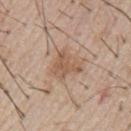follow-up: total-body-photography surveillance lesion; no biopsy
automated metrics: an average lesion color of about L≈56 a*≈18 b*≈30 (CIELAB) and a lesion-to-skin contrast of about 6.5 (normalized; higher = more distinct)
acquisition: ~15 mm tile from a whole-body skin photo
tile lighting: white-light illumination
patient: male, roughly 55 years of age
diameter: about 3.5 mm